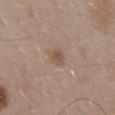Clinical impression: The lesion was photographed on a routine skin check and not biopsied; there is no pathology result. Clinical summary: The lesion-visualizer software estimated an average lesion color of about L≈52 a*≈18 b*≈28 (CIELAB), a lesion–skin lightness drop of about 8, and a normalized border contrast of about 7. Located on the mid back. Captured under white-light illumination. Longest diameter approximately 2.5 mm. A male patient, aged approximately 55. Cropped from a whole-body photographic skin survey; the tile spans about 15 mm.